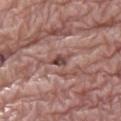Located on the right thigh. The subject is a female in their 70s. This is a white-light tile. The lesion-visualizer software estimated a mean CIELAB color near L≈46 a*≈21 b*≈22, about 12 CIELAB-L* units darker than the surrounding skin, and a normalized lesion–skin contrast near 8.5. It also reported a border-irregularity rating of about 3.5/10, internal color variation of about 3.5 on a 0–10 scale, and peripheral color asymmetry of about 1. A close-up tile cropped from a whole-body skin photograph, about 15 mm across. The lesion's longest dimension is about 3 mm.